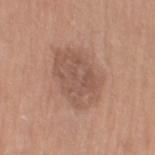biopsy status: imaged on a skin check; not biopsied
patient: female, roughly 55 years of age
size: about 6.5 mm
acquisition: total-body-photography crop, ~15 mm field of view
location: the left thigh
TBP lesion metrics: an average lesion color of about L≈54 a*≈19 b*≈27 (CIELAB), a lesion–skin lightness drop of about 9, and a lesion-to-skin contrast of about 6 (normalized; higher = more distinct); a classifier nevus-likeness of about 10/100 and lesion-presence confidence of about 100/100